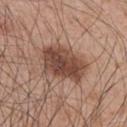workup: imaged on a skin check; not biopsied
diameter: ≈5.5 mm
anatomic site: the left upper arm
subject: male, aged approximately 45
illumination: white-light illumination
image-analysis metrics: a lesion color around L≈45 a*≈21 b*≈26 in CIELAB, about 14 CIELAB-L* units darker than the surrounding skin, and a normalized border contrast of about 10.5; a border-irregularity index near 2.5/10 and a within-lesion color-variation index near 5/10
image source: ~15 mm tile from a whole-body skin photo